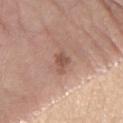Imaged during a routine full-body skin examination; the lesion was not biopsied and no histopathology is available. The lesion is located on the right forearm. About 3 mm across. A male patient aged approximately 70. A region of skin cropped from a whole-body photographic capture, roughly 15 mm wide. Imaged with white-light lighting.